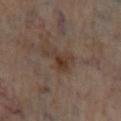{
  "biopsy_status": "not biopsied; imaged during a skin examination",
  "patient": {
    "sex": "male",
    "age_approx": 70
  },
  "lesion_size": {
    "long_diameter_mm_approx": 3.5
  },
  "image": {
    "source": "total-body photography crop",
    "field_of_view_mm": 15
  },
  "automated_metrics": {
    "area_mm2_approx": 5.5,
    "shape_asymmetry": 0.6,
    "cielab_L": 34,
    "cielab_a": 15,
    "cielab_b": 23,
    "vs_skin_darker_L": 7.0,
    "vs_skin_contrast_norm": 7.5
  },
  "site": "left lower leg"
}